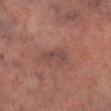Imaged during a routine full-body skin examination; the lesion was not biopsied and no histopathology is available. A male patient approximately 75 years of age. A region of skin cropped from a whole-body photographic capture, roughly 15 mm wide. Located on the right lower leg.